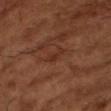workup = no biopsy performed (imaged during a skin exam) | image-analysis metrics = a mean CIELAB color near L≈30 a*≈22 b*≈27, a lesion–skin lightness drop of about 5, and a normalized lesion–skin contrast near 5; a border-irregularity rating of about 4/10, a within-lesion color-variation index near 0/10, and peripheral color asymmetry of about 0 | patient = male, about 65 years old | image = 15 mm crop, total-body photography.